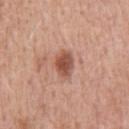Q: Was this lesion biopsied?
A: total-body-photography surveillance lesion; no biopsy
Q: What is the imaging modality?
A: 15 mm crop, total-body photography
Q: Where on the body is the lesion?
A: the chest
Q: Patient demographics?
A: male, aged around 60